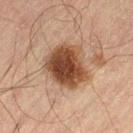No biopsy was performed on this lesion — it was imaged during a full skin examination and was not determined to be concerning.
The lesion is on the left thigh.
A male subject, aged 48–52.
Approximately 5 mm at its widest.
Cropped from a total-body skin-imaging series; the visible field is about 15 mm.
Automated image analysis of the tile measured a classifier nevus-likeness of about 90/100 and lesion-presence confidence of about 100/100.
Captured under cross-polarized illumination.